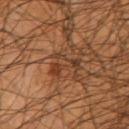Clinical impression:
The lesion was photographed on a routine skin check and not biopsied; there is no pathology result.
Image and clinical context:
The tile uses cross-polarized illumination. The lesion's longest dimension is about 4.5 mm. A region of skin cropped from a whole-body photographic capture, roughly 15 mm wide. A male patient about 45 years old. From the right upper arm.